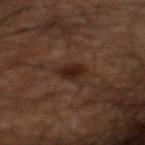Q: Is there a histopathology result?
A: catalogued during a skin exam; not biopsied
Q: Where on the body is the lesion?
A: the mid back
Q: Illumination type?
A: cross-polarized illumination
Q: What kind of image is this?
A: ~15 mm tile from a whole-body skin photo
Q: Patient demographics?
A: male, roughly 60 years of age
Q: How large is the lesion?
A: about 3 mm